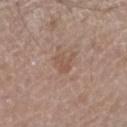Findings:
• workup — total-body-photography surveillance lesion; no biopsy